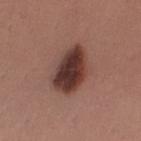The lesion was tiled from a total-body skin photograph and was not biopsied. Longest diameter approximately 5.5 mm. Located on the left upper arm. The tile uses white-light illumination. A male subject, aged 28–32. A region of skin cropped from a whole-body photographic capture, roughly 15 mm wide.